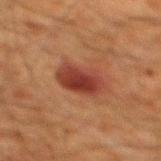Findings:
* follow-up — catalogued during a skin exam; not biopsied
* illumination — cross-polarized illumination
* subject — male, roughly 60 years of age
* diameter — ≈4.5 mm
* image source — ~15 mm crop, total-body skin-cancer survey
* site — the mid back